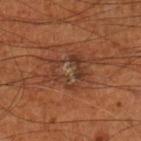The lesion was tiled from a total-body skin photograph and was not biopsied. About 5.5 mm across. Imaged with cross-polarized lighting. The total-body-photography lesion software estimated an outline eccentricity of about 0.7 (0 = round, 1 = elongated) and a symmetry-axis asymmetry near 0.3. The software also gave a border-irregularity index near 5/10, a within-lesion color-variation index near 7.5/10, and radial color variation of about 2.5. The software also gave a classifier nevus-likeness of about 0/100 and a lesion-detection confidence of about 85/100. A 15 mm close-up extracted from a 3D total-body photography capture. Located on the left lower leg. A male subject roughly 70 years of age.